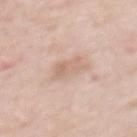Recorded during total-body skin imaging; not selected for excision or biopsy. The recorded lesion diameter is about 3.5 mm. This is a white-light tile. A 15 mm close-up tile from a total-body photography series done for melanoma screening. A female subject aged around 65. An algorithmic analysis of the crop reported an area of roughly 6 mm², an eccentricity of roughly 0.75, and a shape-asymmetry score of about 0.3 (0 = symmetric). The software also gave a border-irregularity index near 3.5/10, a within-lesion color-variation index near 3/10, and peripheral color asymmetry of about 1. The software also gave a nevus-likeness score of about 0/100 and lesion-presence confidence of about 100/100. From the mid back.